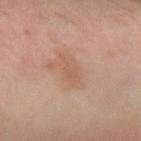Captured during whole-body skin photography for melanoma surveillance; the lesion was not biopsied.
From the arm.
A female patient aged 58 to 62.
Longest diameter approximately 3 mm.
Cropped from a whole-body photographic skin survey; the tile spans about 15 mm.